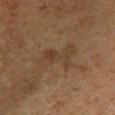Q: Is there a histopathology result?
A: total-body-photography surveillance lesion; no biopsy